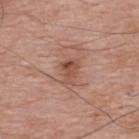follow-up: imaged on a skin check; not biopsied
location: the back
image source: ~15 mm tile from a whole-body skin photo
automated lesion analysis: a mean CIELAB color near L≈50 a*≈23 b*≈28, a lesion–skin lightness drop of about 10, and a lesion-to-skin contrast of about 7 (normalized; higher = more distinct); border irregularity of about 4 on a 0–10 scale, a within-lesion color-variation index near 4.5/10, and peripheral color asymmetry of about 2
lesion size: ≈3 mm
patient: male, aged around 70
lighting: white-light illumination